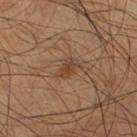Captured during whole-body skin photography for melanoma surveillance; the lesion was not biopsied. A male patient approximately 60 years of age. The tile uses cross-polarized illumination. The lesion is located on the right lower leg. A lesion tile, about 15 mm wide, cut from a 3D total-body photograph.